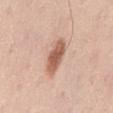Notes:
- workup · catalogued during a skin exam; not biopsied
- illumination · white-light illumination
- patient · male, approximately 40 years of age
- acquisition · 15 mm crop, total-body photography
- site · the mid back